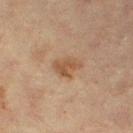workup = total-body-photography surveillance lesion; no biopsy | automated metrics = an average lesion color of about L≈45 a*≈17 b*≈30 (CIELAB), roughly 8 lightness units darker than nearby skin, and a normalized lesion–skin contrast near 7; border irregularity of about 3 on a 0–10 scale and peripheral color asymmetry of about 0.5; a classifier nevus-likeness of about 5/100 and a lesion-detection confidence of about 100/100 | image source = ~15 mm tile from a whole-body skin photo | location = the right thigh | patient = female, in their mid-50s | tile lighting = cross-polarized illumination.